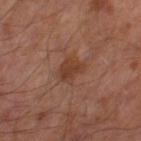notes: imaged on a skin check; not biopsied | anatomic site: the leg | subject: male, about 65 years old | image source: 15 mm crop, total-body photography | lighting: cross-polarized.